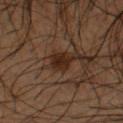- biopsy status — catalogued during a skin exam; not biopsied
- image source — ~15 mm tile from a whole-body skin photo
- anatomic site — the left forearm
- subject — male, approximately 50 years of age
- illumination — cross-polarized illumination
- size — about 2.5 mm